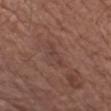Imaged during a routine full-body skin examination; the lesion was not biopsied and no histopathology is available.
A 15 mm close-up extracted from a 3D total-body photography capture.
A male subject, in their mid-70s.
An algorithmic analysis of the crop reported a lesion area of about 3 mm², an eccentricity of roughly 0.95, and a shape-asymmetry score of about 0.45 (0 = symmetric). And it measured border irregularity of about 5.5 on a 0–10 scale, a within-lesion color-variation index near 0/10, and a peripheral color-asymmetry measure near 0.
Located on the left upper arm.